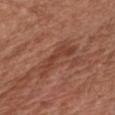Imaged during a routine full-body skin examination; the lesion was not biopsied and no histopathology is available.
The patient is a female aged 73 to 77.
Captured under white-light illumination.
From the chest.
The total-body-photography lesion software estimated an outline eccentricity of about 0.95 (0 = round, 1 = elongated) and a symmetry-axis asymmetry near 0.5. And it measured an automated nevus-likeness rating near 0 out of 100.
Approximately 5.5 mm at its widest.
A lesion tile, about 15 mm wide, cut from a 3D total-body photograph.